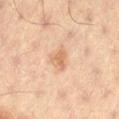{
  "site": "left thigh",
  "image": {
    "source": "total-body photography crop",
    "field_of_view_mm": 15
  },
  "patient": {
    "sex": "male",
    "age_approx": 60
  }
}